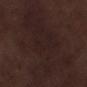Findings:
* imaging modality — ~15 mm crop, total-body skin-cancer survey
* lesion size — ~14.5 mm (longest diameter)
* illumination — white-light
* automated metrics — a lesion color around L≈19 a*≈14 b*≈14 in CIELAB, about 4 CIELAB-L* units darker than the surrounding skin, and a normalized lesion–skin contrast near 5.5; an automated nevus-likeness rating near 0 out of 100 and a lesion-detection confidence of about 65/100
* anatomic site — the left lower leg
* subject — male, roughly 70 years of age